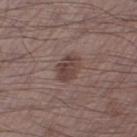Findings:
– workup — no biopsy performed (imaged during a skin exam)
– patient — male, in their 70s
– acquisition — 15 mm crop, total-body photography
– site — the right thigh
– TBP lesion metrics — border irregularity of about 1.5 on a 0–10 scale and a within-lesion color-variation index near 3/10; an automated nevus-likeness rating near 35 out of 100 and a lesion-detection confidence of about 100/100
– lighting — white-light